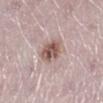Recorded during total-body skin imaging; not selected for excision or biopsy. Measured at roughly 3 mm in maximum diameter. Captured under white-light illumination. A region of skin cropped from a whole-body photographic capture, roughly 15 mm wide. The patient is a female aged around 50. The lesion is on the right lower leg.